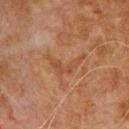Located on the chest. A lesion tile, about 15 mm wide, cut from a 3D total-body photograph. The tile uses cross-polarized illumination. The patient is a male roughly 80 years of age.